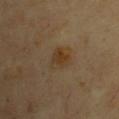Captured under cross-polarized illumination. On the chest. Longest diameter approximately 3 mm. A male subject in their mid-60s. The total-body-photography lesion software estimated roughly 6 lightness units darker than nearby skin and a normalized lesion–skin contrast near 7. The analysis additionally found a border-irregularity rating of about 2.5/10 and radial color variation of about 1.5. The software also gave a classifier nevus-likeness of about 95/100 and a lesion-detection confidence of about 100/100. A roughly 15 mm field-of-view crop from a total-body skin photograph.